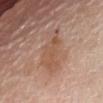Imaged during a routine full-body skin examination; the lesion was not biopsied and no histopathology is available. Located on the abdomen. A female patient, aged 73–77. The recorded lesion diameter is about 5.5 mm. An algorithmic analysis of the crop reported a lesion area of about 12 mm² and a shape-asymmetry score of about 0.35 (0 = symmetric). It also reported a nevus-likeness score of about 25/100 and a detector confidence of about 100 out of 100 that the crop contains a lesion. A 15 mm close-up tile from a total-body photography series done for melanoma screening.